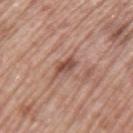Assessment: The lesion was photographed on a routine skin check and not biopsied; there is no pathology result. Context: A lesion tile, about 15 mm wide, cut from a 3D total-body photograph. From the left upper arm. A male patient roughly 70 years of age.